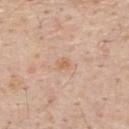The lesion was tiled from a total-body skin photograph and was not biopsied. A 15 mm close-up tile from a total-body photography series done for melanoma screening. From the upper back. The patient is a male aged 53 to 57.